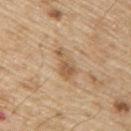notes=total-body-photography surveillance lesion; no biopsy
illumination=white-light
site=the upper back
image=total-body-photography crop, ~15 mm field of view
subject=male, aged 68–72
lesion diameter=about 4 mm
automated lesion analysis=a mean CIELAB color near L≈57 a*≈18 b*≈35, roughly 10 lightness units darker than nearby skin, and a normalized lesion–skin contrast near 7; a peripheral color-asymmetry measure near 1